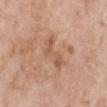  biopsy_status: not biopsied; imaged during a skin examination
  image:
    source: total-body photography crop
    field_of_view_mm: 15
  patient:
    sex: female
    age_approx: 70
  automated_metrics:
    nevus_likeness_0_100: 0
    lesion_detection_confidence_0_100: 100
  lesion_size:
    long_diameter_mm_approx: 4.5
  site: left lower leg
  lighting: white-light